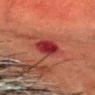Findings:
– workup — no biopsy performed (imaged during a skin exam)
– acquisition — total-body-photography crop, ~15 mm field of view
– tile lighting — cross-polarized illumination
– location — the head or neck
– lesion diameter — ~3.5 mm (longest diameter)
– patient — male, aged 48 to 52